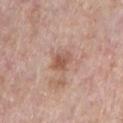Clinical impression: No biopsy was performed on this lesion — it was imaged during a full skin examination and was not determined to be concerning. Image and clinical context: A lesion tile, about 15 mm wide, cut from a 3D total-body photograph. Located on the chest. A male patient, roughly 70 years of age.